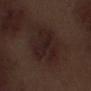The lesion was tiled from a total-body skin photograph and was not biopsied.
A close-up tile cropped from a whole-body skin photograph, about 15 mm across.
Captured under white-light illumination.
Located on the left thigh.
Automated image analysis of the tile measured a footprint of about 22 mm², a shape eccentricity near 0.6, and a shape-asymmetry score of about 0.2 (0 = symmetric). The software also gave a lesion color around L≈20 a*≈15 b*≈16 in CIELAB, roughly 5 lightness units darker than nearby skin, and a normalized border contrast of about 7.5.
The subject is a male roughly 70 years of age.